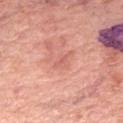{
  "site": "right forearm",
  "patient": {
    "sex": "female",
    "age_approx": 55
  },
  "image": {
    "source": "total-body photography crop",
    "field_of_view_mm": 15
  }
}